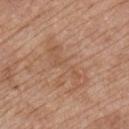{
  "biopsy_status": "not biopsied; imaged during a skin examination",
  "lighting": "white-light",
  "patient": {
    "sex": "male",
    "age_approx": 65
  },
  "image": {
    "source": "total-body photography crop",
    "field_of_view_mm": 15
  },
  "lesion_size": {
    "long_diameter_mm_approx": 7.0
  },
  "automated_metrics": {
    "cielab_L": 55,
    "cielab_a": 20,
    "cielab_b": 31,
    "vs_skin_darker_L": 6.0,
    "vs_skin_contrast_norm": 4.5,
    "nevus_likeness_0_100": 0,
    "lesion_detection_confidence_0_100": 100
  },
  "site": "front of the torso"
}